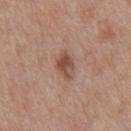Impression: The lesion was tiled from a total-body skin photograph and was not biopsied. Background: A close-up tile cropped from a whole-body skin photograph, about 15 mm across. Measured at roughly 3 mm in maximum diameter. A male patient in their 50s. The lesion is located on the abdomen.